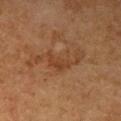notes = imaged on a skin check; not biopsied
lesion diameter = about 6 mm
lighting = cross-polarized
location = the right upper arm
patient = female, approximately 60 years of age
image = ~15 mm tile from a whole-body skin photo